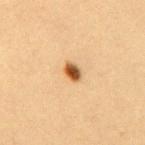Captured under cross-polarized illumination. A female patient, about 30 years old. The lesion is on the upper back. This image is a 15 mm lesion crop taken from a total-body photograph.